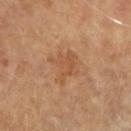biopsy status = imaged on a skin check; not biopsied | location = the arm | lesion size = ≈4 mm | acquisition = 15 mm crop, total-body photography | illumination = cross-polarized | image-analysis metrics = a classifier nevus-likeness of about 0/100 and a lesion-detection confidence of about 100/100 | subject = in their 60s.